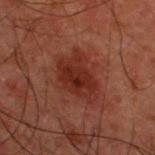| feature | finding |
|---|---|
| notes | catalogued during a skin exam; not biopsied |
| subject | male, aged 48 to 52 |
| location | the upper back |
| size | ≈5 mm |
| image-analysis metrics | a lesion area of about 14 mm², an eccentricity of roughly 0.7, and a symmetry-axis asymmetry near 0.3 |
| illumination | cross-polarized |
| image | ~15 mm tile from a whole-body skin photo |